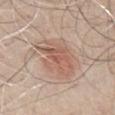Q: Was this lesion biopsied?
A: catalogued during a skin exam; not biopsied
Q: What did automated image analysis measure?
A: a lesion area of about 15 mm², an outline eccentricity of about 0.85 (0 = round, 1 = elongated), and a symmetry-axis asymmetry near 0.25; a border-irregularity index near 3.5/10, a within-lesion color-variation index near 5.5/10, and a peripheral color-asymmetry measure near 2; an automated nevus-likeness rating near 100 out of 100 and lesion-presence confidence of about 100/100
Q: Patient demographics?
A: male, aged 53 to 57
Q: What kind of image is this?
A: total-body-photography crop, ~15 mm field of view
Q: Where on the body is the lesion?
A: the chest
Q: How was the tile lit?
A: white-light illumination